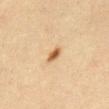follow-up — imaged on a skin check; not biopsied
patient — female, in their mid- to late 60s
imaging modality — total-body-photography crop, ~15 mm field of view
automated lesion analysis — an average lesion color of about L≈52 a*≈18 b*≈35 (CIELAB), a lesion–skin lightness drop of about 13, and a lesion-to-skin contrast of about 9.5 (normalized; higher = more distinct)
lesion diameter — about 2.5 mm
site — the abdomen